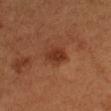Assessment:
Part of a total-body skin-imaging series; this lesion was reviewed on a skin check and was not flagged for biopsy.
Context:
The lesion-visualizer software estimated an area of roughly 5 mm², an eccentricity of roughly 0.65, and two-axis asymmetry of about 0.3. The analysis additionally found about 8 CIELAB-L* units darker than the surrounding skin and a normalized border contrast of about 8. The analysis additionally found a classifier nevus-likeness of about 90/100 and a lesion-detection confidence of about 100/100. Captured under cross-polarized illumination. A female patient aged around 40. A close-up tile cropped from a whole-body skin photograph, about 15 mm across. The recorded lesion diameter is about 3 mm. From the head or neck.